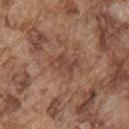The lesion-visualizer software estimated a lesion area of about 6 mm². The software also gave a color-variation rating of about 3.5/10 and radial color variation of about 1. And it measured a nevus-likeness score of about 0/100 and lesion-presence confidence of about 100/100. On the right upper arm. This is a white-light tile. Cropped from a whole-body photographic skin survey; the tile spans about 15 mm. A male patient about 75 years old.